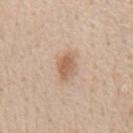Clinical impression:
Imaged during a routine full-body skin examination; the lesion was not biopsied and no histopathology is available.
Context:
A roughly 15 mm field-of-view crop from a total-body skin photograph. A male patient aged around 60. The lesion is located on the mid back.